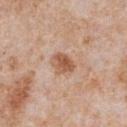Q: Was this lesion biopsied?
A: catalogued during a skin exam; not biopsied
Q: Where on the body is the lesion?
A: the chest
Q: What are the patient's age and sex?
A: male, approximately 65 years of age
Q: How was this image acquired?
A: 15 mm crop, total-body photography